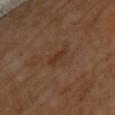Imaged during a routine full-body skin examination; the lesion was not biopsied and no histopathology is available. An algorithmic analysis of the crop reported a lesion color around L≈34 a*≈19 b*≈31 in CIELAB and a lesion-to-skin contrast of about 6.5 (normalized; higher = more distinct). It also reported border irregularity of about 2 on a 0–10 scale, internal color variation of about 0 on a 0–10 scale, and peripheral color asymmetry of about 0. On the chest. The patient is female. The tile uses cross-polarized illumination. A close-up tile cropped from a whole-body skin photograph, about 15 mm across. Longest diameter approximately 2.5 mm.